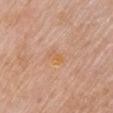No biopsy was performed on this lesion — it was imaged during a full skin examination and was not determined to be concerning. A region of skin cropped from a whole-body photographic capture, roughly 15 mm wide. Located on the chest. The patient is a female aged approximately 60.